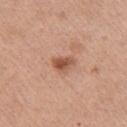<tbp_lesion>
  <biopsy_status>not biopsied; imaged during a skin examination</biopsy_status>
  <patient>
    <sex>male</sex>
    <age_approx>60</age_approx>
  </patient>
  <lighting>white-light</lighting>
  <lesion_size>
    <long_diameter_mm_approx>2.5</long_diameter_mm_approx>
  </lesion_size>
  <site>right upper arm</site>
  <image>
    <source>total-body photography crop</source>
    <field_of_view_mm>15</field_of_view_mm>
  </image>
</tbp_lesion>